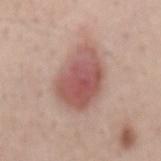The lesion was photographed on a routine skin check and not biopsied; there is no pathology result.
A male patient aged around 40.
Automated image analysis of the tile measured about 13 CIELAB-L* units darker than the surrounding skin and a lesion-to-skin contrast of about 8.5 (normalized; higher = more distinct). It also reported border irregularity of about 2 on a 0–10 scale, a within-lesion color-variation index near 5.5/10, and a peripheral color-asymmetry measure near 1.5. The software also gave a nevus-likeness score of about 100/100.
About 6.5 mm across.
Imaged with white-light lighting.
A region of skin cropped from a whole-body photographic capture, roughly 15 mm wide.
From the lower back.